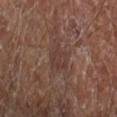Findings:
• imaging modality · ~15 mm crop, total-body skin-cancer survey
• body site · the right forearm
• tile lighting · cross-polarized
• automated lesion analysis · a lesion area of about 5.5 mm² and two-axis asymmetry of about 0.25; an average lesion color of about L≈36 a*≈17 b*≈22 (CIELAB) and a normalized border contrast of about 5
• lesion diameter · ≈3 mm
• subject · male, aged 63–67